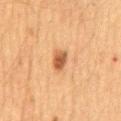Findings:
• workup · no biopsy performed (imaged during a skin exam)
• image · ~15 mm crop, total-body skin-cancer survey
• patient · male, roughly 65 years of age
• body site · the mid back
• TBP lesion metrics · a mean CIELAB color near L≈48 a*≈21 b*≈34, a lesion–skin lightness drop of about 12, and a normalized lesion–skin contrast near 9
• lesion size · ~3 mm (longest diameter)
• illumination · cross-polarized